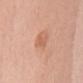<record>
<biopsy_status>not biopsied; imaged during a skin examination</biopsy_status>
<lighting>white-light</lighting>
<lesion_size>
  <long_diameter_mm_approx>3.0</long_diameter_mm_approx>
</lesion_size>
<automated_metrics>
  <area_mm2_approx>3.5</area_mm2_approx>
  <eccentricity>0.85</eccentricity>
  <shape_asymmetry>0.25</shape_asymmetry>
  <cielab_L>61</cielab_L>
  <cielab_a>24</cielab_a>
  <cielab_b>33</cielab_b>
  <vs_skin_darker_L>7.0</vs_skin_darker_L>
  <vs_skin_contrast_norm>5.0</vs_skin_contrast_norm>
  <nevus_likeness_0_100>40</nevus_likeness_0_100>
  <lesion_detection_confidence_0_100>100</lesion_detection_confidence_0_100>
</automated_metrics>
<image>
  <source>total-body photography crop</source>
  <field_of_view_mm>15</field_of_view_mm>
</image>
<site>left upper arm</site>
<patient>
  <sex>female</sex>
  <age_approx>50</age_approx>
</patient>
</record>